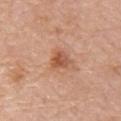Part of a total-body skin-imaging series; this lesion was reviewed on a skin check and was not flagged for biopsy. A roughly 15 mm field-of-view crop from a total-body skin photograph. Located on the left arm. A female patient aged 63–67. Captured under white-light illumination.